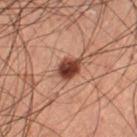follow-up = no biopsy performed (imaged during a skin exam)
anatomic site = the right lower leg
TBP lesion metrics = a lesion color around L≈32 a*≈19 b*≈23 in CIELAB, about 14 CIELAB-L* units darker than the surrounding skin, and a normalized border contrast of about 12.5; a border-irregularity rating of about 1.5/10 and a within-lesion color-variation index near 4/10; an automated nevus-likeness rating near 100 out of 100 and lesion-presence confidence of about 100/100
lesion diameter = ≈3.5 mm
image = ~15 mm tile from a whole-body skin photo
patient = male, about 60 years old